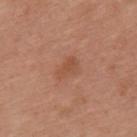biopsy status=imaged on a skin check; not biopsied
subject=female, in their 40s
location=the back
image source=total-body-photography crop, ~15 mm field of view